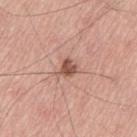| key | value |
|---|---|
| notes | catalogued during a skin exam; not biopsied |
| automated metrics | a lesion–skin lightness drop of about 13 and a lesion-to-skin contrast of about 8.5 (normalized; higher = more distinct); a border-irregularity rating of about 2.5/10, internal color variation of about 2.5 on a 0–10 scale, and radial color variation of about 1 |
| lighting | white-light |
| location | the left thigh |
| patient | male, about 70 years old |
| image | 15 mm crop, total-body photography |
| lesion diameter | ≈2.5 mm |